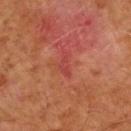No biopsy was performed on this lesion — it was imaged during a full skin examination and was not determined to be concerning. Cropped from a total-body skin-imaging series; the visible field is about 15 mm. On the arm. Imaged with cross-polarized lighting. A male subject aged 68–72. Automated tile analysis of the lesion measured a mean CIELAB color near L≈36 a*≈30 b*≈25 and roughly 5 lightness units darker than nearby skin. It also reported a nevus-likeness score of about 0/100.